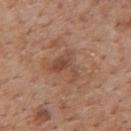The lesion was photographed on a routine skin check and not biopsied; there is no pathology result. The lesion is on the mid back. A 15 mm close-up extracted from a 3D total-body photography capture. The tile uses white-light illumination. Measured at roughly 4 mm in maximum diameter. The patient is a male aged around 60. Automated tile analysis of the lesion measured an automated nevus-likeness rating near 5 out of 100 and a detector confidence of about 100 out of 100 that the crop contains a lesion.